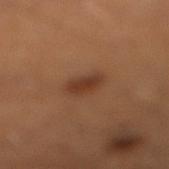This lesion was catalogued during total-body skin photography and was not selected for biopsy.
A lesion tile, about 15 mm wide, cut from a 3D total-body photograph.
The tile uses cross-polarized illumination.
From the right lower leg.
Measured at roughly 3 mm in maximum diameter.
The subject is a male aged 38–42.
Automated tile analysis of the lesion measured a lesion color around L≈37 a*≈22 b*≈31 in CIELAB, a lesion–skin lightness drop of about 9, and a normalized border contrast of about 7.5.